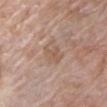Findings:
• biopsy status · imaged on a skin check; not biopsied